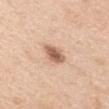Clinical impression:
Imaged during a routine full-body skin examination; the lesion was not biopsied and no histopathology is available.
Background:
A male patient aged around 60. The lesion's longest dimension is about 3.5 mm. The total-body-photography lesion software estimated a lesion color around L≈61 a*≈21 b*≈31 in CIELAB, a lesion–skin lightness drop of about 15, and a normalized border contrast of about 9. It also reported an automated nevus-likeness rating near 90 out of 100 and a detector confidence of about 100 out of 100 that the crop contains a lesion. The tile uses white-light illumination. A 15 mm close-up extracted from a 3D total-body photography capture. The lesion is located on the left upper arm.